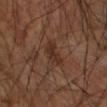{"biopsy_status": "not biopsied; imaged during a skin examination", "automated_metrics": {"area_mm2_approx": 4.5, "eccentricity": 0.75, "border_irregularity_0_10": 4.0, "color_variation_0_10": 1.5}, "image": {"source": "total-body photography crop", "field_of_view_mm": 15}, "lighting": "cross-polarized", "patient": {"sex": "male", "age_approx": 65}, "site": "left forearm", "lesion_size": {"long_diameter_mm_approx": 3.0}}